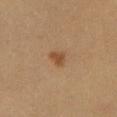Cropped from a whole-body photographic skin survey; the tile spans about 15 mm.
The lesion is located on the chest.
The subject is a male aged approximately 20.
Automated tile analysis of the lesion measured a footprint of about 3 mm² and two-axis asymmetry of about 0.3. The analysis additionally found a color-variation rating of about 1.5/10.
The tile uses cross-polarized illumination.